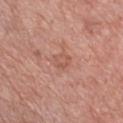Case summary:
* biopsy status: imaged on a skin check; not biopsied
* image source: ~15 mm tile from a whole-body skin photo
* lighting: white-light
* anatomic site: the chest
* subject: male, aged approximately 50
* size: ≈2.5 mm
* automated lesion analysis: a lesion area of about 4.5 mm² and a symmetry-axis asymmetry near 0.25; a mean CIELAB color near L≈56 a*≈24 b*≈29, about 6 CIELAB-L* units darker than the surrounding skin, and a lesion-to-skin contrast of about 4.5 (normalized; higher = more distinct)